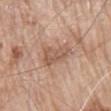| key | value |
|---|---|
| notes | imaged on a skin check; not biopsied |
| patient | male, aged 78 to 82 |
| anatomic site | the back |
| acquisition | ~15 mm tile from a whole-body skin photo |
| illumination | white-light |